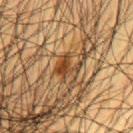Part of a total-body skin-imaging series; this lesion was reviewed on a skin check and was not flagged for biopsy.
The tile uses cross-polarized illumination.
Located on the upper back.
The recorded lesion diameter is about 3.5 mm.
A male patient, roughly 60 years of age.
This image is a 15 mm lesion crop taken from a total-body photograph.